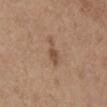follow-up = imaged on a skin check; not biopsied
tile lighting = white-light illumination
image-analysis metrics = an area of roughly 4 mm² and a shape eccentricity near 0.9; an average lesion color of about L≈50 a*≈18 b*≈29 (CIELAB), a lesion–skin lightness drop of about 9, and a normalized border contrast of about 6.5
image source = ~15 mm tile from a whole-body skin photo
patient = female, about 65 years old
anatomic site = the chest
lesion size = ~3.5 mm (longest diameter)